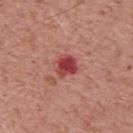This lesion was catalogued during total-body skin photography and was not selected for biopsy.
The tile uses white-light illumination.
A male patient, about 70 years old.
From the upper back.
A 15 mm close-up extracted from a 3D total-body photography capture.
The total-body-photography lesion software estimated a lesion area of about 6 mm² and a shape eccentricity near 0.35. The software also gave a lesion color around L≈46 a*≈35 b*≈24 in CIELAB, about 13 CIELAB-L* units darker than the surrounding skin, and a lesion-to-skin contrast of about 9.5 (normalized; higher = more distinct). And it measured a classifier nevus-likeness of about 0/100 and a lesion-detection confidence of about 100/100.
The recorded lesion diameter is about 2.5 mm.